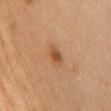The lesion was photographed on a routine skin check and not biopsied; there is no pathology result. Cropped from a whole-body photographic skin survey; the tile spans about 15 mm. A female patient aged 58 to 62. The lesion is on the front of the torso.